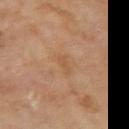From the right upper arm.
The lesion's longest dimension is about 2.5 mm.
A male subject in their 70s.
The lesion-visualizer software estimated a lesion color around L≈52 a*≈19 b*≈35 in CIELAB, roughly 5 lightness units darker than nearby skin, and a lesion-to-skin contrast of about 4.5 (normalized; higher = more distinct). The analysis additionally found a border-irregularity rating of about 3.5/10, internal color variation of about 0 on a 0–10 scale, and peripheral color asymmetry of about 0. The software also gave a lesion-detection confidence of about 100/100.
The tile uses cross-polarized illumination.
A 15 mm crop from a total-body photograph taken for skin-cancer surveillance.